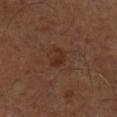• workup · no biopsy performed (imaged during a skin exam)
• illumination · cross-polarized
• automated lesion analysis · a border-irregularity rating of about 4.5/10, internal color variation of about 0.5 on a 0–10 scale, and a peripheral color-asymmetry measure near 0; an automated nevus-likeness rating near 5 out of 100 and a detector confidence of about 100 out of 100 that the crop contains a lesion
• patient · aged 63 to 67
• body site · the left lower leg
• acquisition · ~15 mm crop, total-body skin-cancer survey
• lesion size · ~2.5 mm (longest diameter)